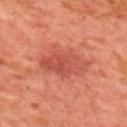<record>
  <automated_metrics>
    <cielab_L>52</cielab_L>
    <cielab_a>33</cielab_a>
    <cielab_b>32</cielab_b>
    <vs_skin_darker_L>9.0</vs_skin_darker_L>
    <vs_skin_contrast_norm>6.0</vs_skin_contrast_norm>
    <border_irregularity_0_10>3.0</border_irregularity_0_10>
    <color_variation_0_10>4.5</color_variation_0_10>
    <peripheral_color_asymmetry>1.5</peripheral_color_asymmetry>
  </automated_metrics>
  <lighting>cross-polarized</lighting>
  <image>
    <source>total-body photography crop</source>
    <field_of_view_mm>15</field_of_view_mm>
  </image>
  <patient>
    <sex>male</sex>
    <age_approx>65</age_approx>
  </patient>
  <lesion_size>
    <long_diameter_mm_approx>5.5</long_diameter_mm_approx>
  </lesion_size>
  <site>upper back</site>
</record>